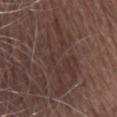{"biopsy_status": "not biopsied; imaged during a skin examination", "lesion_size": {"long_diameter_mm_approx": 10.0}, "patient": {"sex": "female", "age_approx": 75}, "image": {"source": "total-body photography crop", "field_of_view_mm": 15}, "automated_metrics": {"cielab_L": 34, "cielab_a": 16, "cielab_b": 19, "vs_skin_darker_L": 6.0, "vs_skin_contrast_norm": 5.5, "border_irregularity_0_10": 7.5, "color_variation_0_10": 3.5, "peripheral_color_asymmetry": 1.5, "nevus_likeness_0_100": 0, "lesion_detection_confidence_0_100": 80}, "lighting": "white-light", "site": "head or neck"}